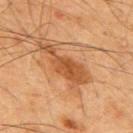Captured during whole-body skin photography for melanoma surveillance; the lesion was not biopsied. Approximately 7 mm at its widest. Located on the upper back. Captured under cross-polarized illumination. The subject is a male about 65 years old. A 15 mm crop from a total-body photograph taken for skin-cancer surveillance.